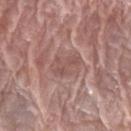This lesion was catalogued during total-body skin photography and was not selected for biopsy.
The lesion-visualizer software estimated an average lesion color of about L≈51 a*≈21 b*≈22 (CIELAB) and a normalized lesion–skin contrast near 5.5. The analysis additionally found border irregularity of about 8.5 on a 0–10 scale, a color-variation rating of about 0.5/10, and peripheral color asymmetry of about 0.
The lesion is on the left forearm.
This is a white-light tile.
A male subject, aged around 80.
A roughly 15 mm field-of-view crop from a total-body skin photograph.